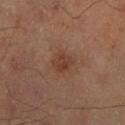| field | value |
|---|---|
| workup | imaged on a skin check; not biopsied |
| site | the right lower leg |
| automated lesion analysis | an area of roughly 5 mm² and a shape-asymmetry score of about 0.25 (0 = symmetric); a mean CIELAB color near L≈38 a*≈20 b*≈28 and a lesion–skin lightness drop of about 7; a border-irregularity index near 2/10 and a within-lesion color-variation index near 2.5/10; lesion-presence confidence of about 100/100 |
| illumination | cross-polarized |
| patient | male, aged 58 to 62 |
| lesion diameter | about 2.5 mm |
| image source | ~15 mm crop, total-body skin-cancer survey |